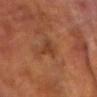| key | value |
|---|---|
| follow-up | total-body-photography surveillance lesion; no biopsy |
| anatomic site | the upper back |
| subject | male, in their 60s |
| imaging modality | total-body-photography crop, ~15 mm field of view |